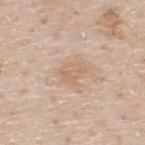workup = no biopsy performed (imaged during a skin exam)
lesion diameter = about 3 mm
site = the upper back
subject = male, aged approximately 80
tile lighting = white-light
image source = ~15 mm tile from a whole-body skin photo